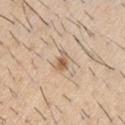Imaged during a routine full-body skin examination; the lesion was not biopsied and no histopathology is available. On the right upper arm. A 15 mm crop from a total-body photograph taken for skin-cancer surveillance. A male patient aged approximately 60. About 2.5 mm across.